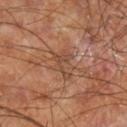workup — no biopsy performed (imaged during a skin exam); tile lighting — cross-polarized illumination; location — the right lower leg; subject — male, aged 58 to 62; diameter — about 3 mm; imaging modality — total-body-photography crop, ~15 mm field of view.